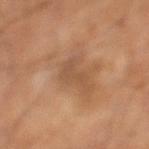lighting = cross-polarized illumination; imaging modality = total-body-photography crop, ~15 mm field of view; body site = the left lower leg; lesion size = about 5 mm; subject = male, about 65 years old.